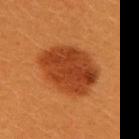Background:
The subject is a female aged 28–32. The lesion is on the upper back. Measured at roughly 7 mm in maximum diameter. The tile uses cross-polarized illumination. A close-up tile cropped from a whole-body skin photograph, about 15 mm across. The lesion-visualizer software estimated a shape eccentricity near 0.65 and a shape-asymmetry score of about 0.15 (0 = symmetric). And it measured border irregularity of about 1.5 on a 0–10 scale and internal color variation of about 4 on a 0–10 scale.